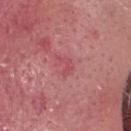| key | value |
|---|---|
| follow-up | no biopsy performed (imaged during a skin exam) |
| lesion size | about 2.5 mm |
| subject | male, roughly 55 years of age |
| automated metrics | a footprint of about 3 mm², an outline eccentricity of about 0.8 (0 = round, 1 = elongated), and two-axis asymmetry of about 0.7; a classifier nevus-likeness of about 0/100 and lesion-presence confidence of about 95/100 |
| image | total-body-photography crop, ~15 mm field of view |
| anatomic site | the head or neck |
| tile lighting | white-light illumination |